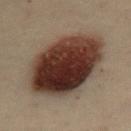Assessment:
The lesion was photographed on a routine skin check and not biopsied; there is no pathology result.
Clinical summary:
A female patient about 30 years old. The lesion is located on the abdomen. A region of skin cropped from a whole-body photographic capture, roughly 15 mm wide. The tile uses cross-polarized illumination.